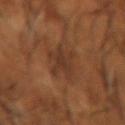No biopsy was performed on this lesion — it was imaged during a full skin examination and was not determined to be concerning.
The lesion's longest dimension is about 5 mm.
The subject is a male in their mid- to late 60s.
Automated tile analysis of the lesion measured a footprint of about 9 mm², an outline eccentricity of about 0.7 (0 = round, 1 = elongated), and two-axis asymmetry of about 0.35. It also reported a lesion color around L≈35 a*≈20 b*≈29 in CIELAB, about 6 CIELAB-L* units darker than the surrounding skin, and a normalized lesion–skin contrast near 6.5. It also reported internal color variation of about 2.5 on a 0–10 scale and peripheral color asymmetry of about 1. The analysis additionally found a classifier nevus-likeness of about 0/100 and a detector confidence of about 95 out of 100 that the crop contains a lesion.
A close-up tile cropped from a whole-body skin photograph, about 15 mm across.
The tile uses cross-polarized illumination.
The lesion is on the left forearm.